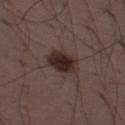The lesion was tiled from a total-body skin photograph and was not biopsied.
This is a white-light tile.
Located on the left thigh.
A male subject aged 48 to 52.
A 15 mm close-up extracted from a 3D total-body photography capture.